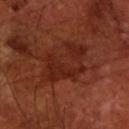Part of a total-body skin-imaging series; this lesion was reviewed on a skin check and was not flagged for biopsy.
The subject is a male aged approximately 70.
Cropped from a whole-body photographic skin survey; the tile spans about 15 mm.
The total-body-photography lesion software estimated a mean CIELAB color near L≈25 a*≈26 b*≈28, a lesion–skin lightness drop of about 6, and a lesion-to-skin contrast of about 6.5 (normalized; higher = more distinct). It also reported a classifier nevus-likeness of about 0/100.
From the front of the torso.